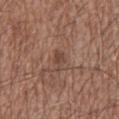Part of a total-body skin-imaging series; this lesion was reviewed on a skin check and was not flagged for biopsy.
This image is a 15 mm lesion crop taken from a total-body photograph.
Automated tile analysis of the lesion measured an outline eccentricity of about 0.85 (0 = round, 1 = elongated) and a shape-asymmetry score of about 0.35 (0 = symmetric). And it measured border irregularity of about 4 on a 0–10 scale and a color-variation rating of about 2/10.
The subject is a male in their mid- to late 70s.
This is a white-light tile.
The lesion is on the mid back.
Longest diameter approximately 3 mm.